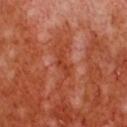Case summary:
- notes · total-body-photography surveillance lesion; no biopsy
- anatomic site · the chest
- patient · male, roughly 55 years of age
- acquisition · total-body-photography crop, ~15 mm field of view
- lighting · cross-polarized
- image-analysis metrics · a lesion area of about 3.5 mm², an outline eccentricity of about 0.95 (0 = round, 1 = elongated), and a shape-asymmetry score of about 0.55 (0 = symmetric); a mean CIELAB color near L≈44 a*≈34 b*≈39, about 7 CIELAB-L* units darker than the surrounding skin, and a normalized lesion–skin contrast near 6.5; a classifier nevus-likeness of about 0/100 and lesion-presence confidence of about 85/100
- diameter · about 3.5 mm